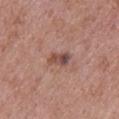workup=catalogued during a skin exam; not biopsied
image source=total-body-photography crop, ~15 mm field of view
subject=male, in their 70s
lighting=white-light illumination
site=the upper back